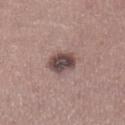Impression: Imaged during a routine full-body skin examination; the lesion was not biopsied and no histopathology is available. Image and clinical context: The tile uses white-light illumination. A 15 mm crop from a total-body photograph taken for skin-cancer surveillance. From the left lower leg. A male patient in their mid- to late 40s.